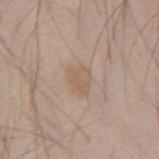biopsy status: no biopsy performed (imaged during a skin exam)
site: the mid back
patient: male, in their mid-40s
image-analysis metrics: a lesion color around L≈59 a*≈15 b*≈28 in CIELAB and roughly 6 lightness units darker than nearby skin; border irregularity of about 1.5 on a 0–10 scale and radial color variation of about 0.5
image: ~15 mm tile from a whole-body skin photo
size: ~3 mm (longest diameter)
lighting: white-light illumination